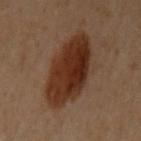This lesion was catalogued during total-body skin photography and was not selected for biopsy.
This image is a 15 mm lesion crop taken from a total-body photograph.
A female subject about 60 years old.
The tile uses cross-polarized illumination.
An algorithmic analysis of the crop reported an area of roughly 30 mm², a shape eccentricity near 0.8, and a symmetry-axis asymmetry near 0.1. And it measured a mean CIELAB color near L≈26 a*≈18 b*≈25, roughly 11 lightness units darker than nearby skin, and a normalized lesion–skin contrast near 11.5. The software also gave a within-lesion color-variation index near 5/10. The software also gave a nevus-likeness score of about 100/100 and lesion-presence confidence of about 100/100.
On the right arm.
About 8 mm across.